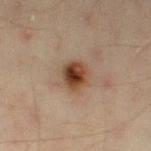Clinical impression:
Recorded during total-body skin imaging; not selected for excision or biopsy.
Clinical summary:
Captured under cross-polarized illumination. The lesion is on the left thigh. Measured at roughly 3 mm in maximum diameter. A 15 mm close-up tile from a total-body photography series done for melanoma screening. The patient is a male in their mid- to late 40s.